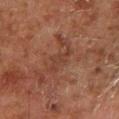biopsy status: total-body-photography surveillance lesion; no biopsy
site: the left lower leg
lighting: cross-polarized illumination
patient: male, in their 80s
automated lesion analysis: an area of roughly 5 mm², an outline eccentricity of about 0.9 (0 = round, 1 = elongated), and a shape-asymmetry score of about 0.45 (0 = symmetric); a mean CIELAB color near L≈30 a*≈18 b*≈23; border irregularity of about 6 on a 0–10 scale and a within-lesion color-variation index near 1.5/10; a nevus-likeness score of about 0/100
imaging modality: ~15 mm crop, total-body skin-cancer survey
lesion diameter: about 3.5 mm